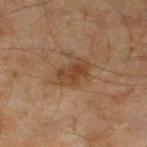Imaged during a routine full-body skin examination; the lesion was not biopsied and no histopathology is available. The lesion is on the left lower leg. The patient is a male about 45 years old. Automated tile analysis of the lesion measured a peripheral color-asymmetry measure near 1. It also reported an automated nevus-likeness rating near 30 out of 100. A 15 mm crop from a total-body photograph taken for skin-cancer surveillance. The tile uses cross-polarized illumination.